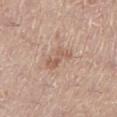follow-up=no biopsy performed (imaged during a skin exam) | illumination=white-light | lesion size=≈3.5 mm | patient=female, aged approximately 65 | TBP lesion metrics=an average lesion color of about L≈58 a*≈19 b*≈28 (CIELAB), roughly 9 lightness units darker than nearby skin, and a normalized lesion–skin contrast near 6; border irregularity of about 5.5 on a 0–10 scale, internal color variation of about 2 on a 0–10 scale, and a peripheral color-asymmetry measure near 0.5 | location=the right lower leg | acquisition=15 mm crop, total-body photography.